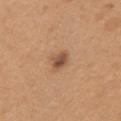The lesion was photographed on a routine skin check and not biopsied; there is no pathology result. From the front of the torso. A lesion tile, about 15 mm wide, cut from a 3D total-body photograph. The subject is a female aged 28 to 32.